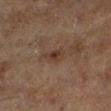Q: Was this lesion biopsied?
A: total-body-photography surveillance lesion; no biopsy
Q: What kind of image is this?
A: ~15 mm tile from a whole-body skin photo
Q: How large is the lesion?
A: about 3 mm
Q: What are the patient's age and sex?
A: male, aged 73–77
Q: What is the anatomic site?
A: the left lower leg